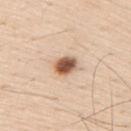<lesion>
<biopsy_status>not biopsied; imaged during a skin examination</biopsy_status>
<lesion_size>
  <long_diameter_mm_approx>3.0</long_diameter_mm_approx>
</lesion_size>
<image>
  <source>total-body photography crop</source>
  <field_of_view_mm>15</field_of_view_mm>
</image>
<lighting>white-light</lighting>
<patient>
  <sex>male</sex>
  <age_approx>60</age_approx>
</patient>
<site>right upper arm</site>
</lesion>